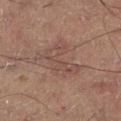Assessment:
Part of a total-body skin-imaging series; this lesion was reviewed on a skin check and was not flagged for biopsy.
Context:
A male patient in their 60s. The lesion is on the left lower leg. Imaged with cross-polarized lighting. A 15 mm close-up tile from a total-body photography series done for melanoma screening.